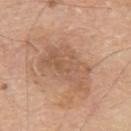No biopsy was performed on this lesion — it was imaged during a full skin examination and was not determined to be concerning. The lesion is located on the upper back. This image is a 15 mm lesion crop taken from a total-body photograph. A male subject, aged around 80. Captured under white-light illumination. Measured at roughly 6.5 mm in maximum diameter.